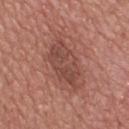Assessment:
Captured during whole-body skin photography for melanoma surveillance; the lesion was not biopsied.
Acquisition and patient details:
The lesion is on the back. A male patient, aged around 60. The tile uses white-light illumination. The total-body-photography lesion software estimated a lesion area of about 15 mm², an outline eccentricity of about 0.85 (0 = round, 1 = elongated), and a symmetry-axis asymmetry near 0.2. The analysis additionally found an average lesion color of about L≈46 a*≈24 b*≈25 (CIELAB), a lesion–skin lightness drop of about 9, and a normalized border contrast of about 7. This image is a 15 mm lesion crop taken from a total-body photograph. Approximately 6.5 mm at its widest.